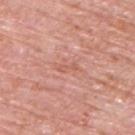{
  "biopsy_status": "not biopsied; imaged during a skin examination",
  "patient": {
    "sex": "male",
    "age_approx": 80
  },
  "image": {
    "source": "total-body photography crop",
    "field_of_view_mm": 15
  },
  "site": "upper back",
  "automated_metrics": {
    "area_mm2_approx": 2.5,
    "eccentricity": 0.9,
    "shape_asymmetry": 0.4,
    "cielab_L": 59,
    "cielab_a": 26,
    "cielab_b": 30,
    "vs_skin_contrast_norm": 4.5,
    "nevus_likeness_0_100": 0,
    "lesion_detection_confidence_0_100": 100
  },
  "lesion_size": {
    "long_diameter_mm_approx": 2.5
  },
  "lighting": "white-light"
}